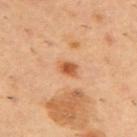notes: total-body-photography surveillance lesion; no biopsy | lighting: cross-polarized | patient: male, about 55 years old | image: ~15 mm tile from a whole-body skin photo | size: ~2.5 mm (longest diameter) | site: the upper back.